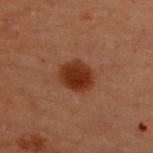Captured during whole-body skin photography for melanoma surveillance; the lesion was not biopsied.
On the back.
A close-up tile cropped from a whole-body skin photograph, about 15 mm across.
This is a cross-polarized tile.
The lesion's longest dimension is about 3.5 mm.
An algorithmic analysis of the crop reported a footprint of about 9 mm², an outline eccentricity of about 0.5 (0 = round, 1 = elongated), and a symmetry-axis asymmetry near 0.15. It also reported a color-variation rating of about 3/10 and a peripheral color-asymmetry measure near 1. It also reported an automated nevus-likeness rating near 100 out of 100 and a detector confidence of about 100 out of 100 that the crop contains a lesion.
A female patient, about 50 years old.